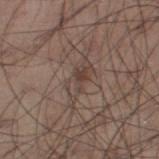biopsy status = catalogued during a skin exam; not biopsied | location = the right thigh | lighting = white-light illumination | subject = male, aged approximately 55 | lesion size = about 4.5 mm | acquisition = total-body-photography crop, ~15 mm field of view | automated metrics = a lesion area of about 7 mm² and a shape eccentricity near 0.9; a mean CIELAB color near L≈42 a*≈14 b*≈21, about 7 CIELAB-L* units darker than the surrounding skin, and a normalized border contrast of about 5.5; a border-irregularity index near 4.5/10, internal color variation of about 7 on a 0–10 scale, and a peripheral color-asymmetry measure near 2.5.